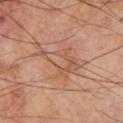The lesion was photographed on a routine skin check and not biopsied; there is no pathology result. On the chest. A 15 mm close-up tile from a total-body photography series done for melanoma screening. The subject is a male approximately 70 years of age.